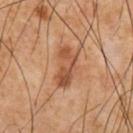No biopsy was performed on this lesion — it was imaged during a full skin examination and was not determined to be concerning.
The tile uses cross-polarized illumination.
From the chest.
The patient is a male aged approximately 65.
A lesion tile, about 15 mm wide, cut from a 3D total-body photograph.